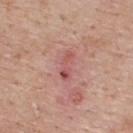Clinical impression: Imaged during a routine full-body skin examination; the lesion was not biopsied and no histopathology is available. Context: The patient is a male aged 58–62. A lesion tile, about 15 mm wide, cut from a 3D total-body photograph. Located on the upper back. Longest diameter approximately 3.5 mm. The tile uses white-light illumination.